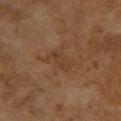This lesion was catalogued during total-body skin photography and was not selected for biopsy.
A 15 mm close-up tile from a total-body photography series done for melanoma screening.
A female patient, aged 58 to 62.
About 3.5 mm across.
Located on the back.
Imaged with cross-polarized lighting.